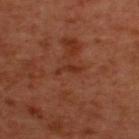Assessment: Imaged during a routine full-body skin examination; the lesion was not biopsied and no histopathology is available. Clinical summary: Located on the upper back. A roughly 15 mm field-of-view crop from a total-body skin photograph. The patient is a male roughly 50 years of age.